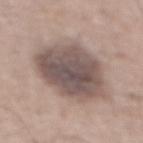Imaged during a routine full-body skin examination; the lesion was not biopsied and no histopathology is available.
The tile uses white-light illumination.
Cropped from a whole-body photographic skin survey; the tile spans about 15 mm.
The subject is a male in their mid-60s.
The lesion is located on the abdomen.